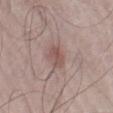Recorded during total-body skin imaging; not selected for excision or biopsy.
An algorithmic analysis of the crop reported a footprint of about 5.5 mm², a shape eccentricity near 0.8, and a shape-asymmetry score of about 0.2 (0 = symmetric). It also reported a mean CIELAB color near L≈52 a*≈18 b*≈21 and a lesion-to-skin contrast of about 6.5 (normalized; higher = more distinct). It also reported a border-irregularity index near 2/10, a color-variation rating of about 3/10, and a peripheral color-asymmetry measure near 1.
Imaged with white-light lighting.
The lesion is on the right lower leg.
Cropped from a whole-body photographic skin survey; the tile spans about 15 mm.
A male subject roughly 50 years of age.
The lesion's longest dimension is about 3.5 mm.